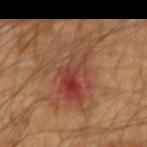From the left forearm.
This is a cross-polarized tile.
Automated image analysis of the tile measured an area of roughly 24 mm², a shape eccentricity near 0.8, and two-axis asymmetry of about 0.45. The analysis additionally found a border-irregularity rating of about 8.5/10, a color-variation rating of about 9.5/10, and peripheral color asymmetry of about 3.5.
The subject is a male aged 48–52.
Cropped from a whole-body photographic skin survey; the tile spans about 15 mm.